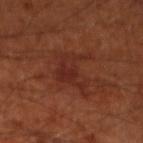An algorithmic analysis of the crop reported a footprint of about 9 mm², an outline eccentricity of about 0.6 (0 = round, 1 = elongated), and a symmetry-axis asymmetry near 0.55. It also reported a border-irregularity rating of about 9/10, a within-lesion color-variation index near 3.5/10, and a peripheral color-asymmetry measure near 1. It also reported an automated nevus-likeness rating near 0 out of 100 and a lesion-detection confidence of about 100/100. Cropped from a whole-body photographic skin survey; the tile spans about 15 mm. The lesion is on the left lower leg. The recorded lesion diameter is about 4.5 mm. A male patient, aged approximately 70.